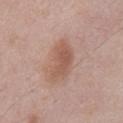Findings:
• workup — imaged on a skin check; not biopsied
• tile lighting — white-light illumination
• location — the abdomen
• subject — male, in their mid- to late 50s
• acquisition — ~15 mm crop, total-body skin-cancer survey
• lesion size — ≈5 mm
• image-analysis metrics — a border-irregularity index near 3.5/10, a within-lesion color-variation index near 2.5/10, and peripheral color asymmetry of about 1